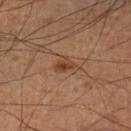Recorded during total-body skin imaging; not selected for excision or biopsy.
The tile uses cross-polarized illumination.
The lesion is located on the leg.
A close-up tile cropped from a whole-body skin photograph, about 15 mm across.
Longest diameter approximately 2.5 mm.
Automated image analysis of the tile measured a border-irregularity index near 3/10, internal color variation of about 3.5 on a 0–10 scale, and peripheral color asymmetry of about 1. It also reported a classifier nevus-likeness of about 85/100 and a lesion-detection confidence of about 100/100.
A male subject aged approximately 60.